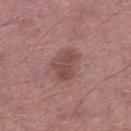patient: male, approximately 55 years of age; lesion diameter: ~4 mm (longest diameter); imaging modality: ~15 mm crop, total-body skin-cancer survey; site: the left lower leg; tile lighting: white-light.